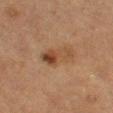follow-up — total-body-photography surveillance lesion; no biopsy | subject — male, approximately 75 years of age | image source — 15 mm crop, total-body photography | lesion size — ≈4.5 mm | illumination — cross-polarized | body site — the left thigh.